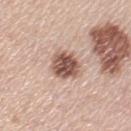Part of a total-body skin-imaging series; this lesion was reviewed on a skin check and was not flagged for biopsy.
About 3.5 mm across.
A female patient, in their 60s.
The lesion is on the left upper arm.
This is a white-light tile.
This image is a 15 mm lesion crop taken from a total-body photograph.